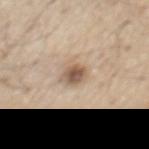Clinical impression: The lesion was photographed on a routine skin check and not biopsied; there is no pathology result. Acquisition and patient details: The recorded lesion diameter is about 3 mm. The lesion is located on the chest. The subject is a male about 70 years old. This is a white-light tile. Automated image analysis of the tile measured a shape eccentricity near 0.7. The software also gave a lesion-detection confidence of about 100/100. A 15 mm close-up tile from a total-body photography series done for melanoma screening.